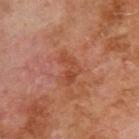This lesion was catalogued during total-body skin photography and was not selected for biopsy. A male subject, aged 68–72. The lesion-visualizer software estimated a lesion color around L≈46 a*≈27 b*≈33 in CIELAB and a normalized border contrast of about 5.5. It also reported a classifier nevus-likeness of about 0/100. Measured at roughly 3.5 mm in maximum diameter. A 15 mm close-up extracted from a 3D total-body photography capture. Located on the upper back. This is a cross-polarized tile.